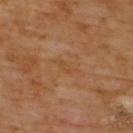Q: Is there a histopathology result?
A: imaged on a skin check; not biopsied
Q: What is the anatomic site?
A: the upper back
Q: Patient demographics?
A: male, aged 58 to 62
Q: How was this image acquired?
A: ~15 mm crop, total-body skin-cancer survey
Q: How was the tile lit?
A: cross-polarized illumination